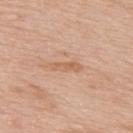The lesion was tiled from a total-body skin photograph and was not biopsied. Longest diameter approximately 3.5 mm. A male patient, aged approximately 80. Cropped from a total-body skin-imaging series; the visible field is about 15 mm. The lesion is located on the back. The tile uses white-light illumination.